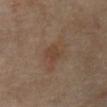<tbp_lesion>
  <biopsy_status>not biopsied; imaged during a skin examination</biopsy_status>
  <lesion_size>
    <long_diameter_mm_approx>2.5</long_diameter_mm_approx>
  </lesion_size>
  <image>
    <source>total-body photography crop</source>
    <field_of_view_mm>15</field_of_view_mm>
  </image>
  <automated_metrics>
    <peripheral_color_asymmetry>1.0</peripheral_color_asymmetry>
  </automated_metrics>
  <lighting>cross-polarized</lighting>
  <site>abdomen</site>
  <patient>
    <sex>male</sex>
    <age_approx>70</age_approx>
  </patient>
</tbp_lesion>